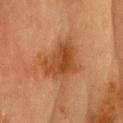Findings:
- biopsy status · imaged on a skin check; not biopsied
- location · the head or neck
- subject · male, roughly 70 years of age
- image source · total-body-photography crop, ~15 mm field of view
- size · ~5.5 mm (longest diameter)
- automated lesion analysis · a shape eccentricity near 0.3 and a shape-asymmetry score of about 0.35 (0 = symmetric); a border-irregularity rating of about 3.5/10 and radial color variation of about 1.5; lesion-presence confidence of about 100/100